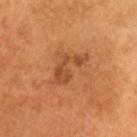workup: imaged on a skin check; not biopsied
patient: male, roughly 60 years of age
lesion diameter: ≈5 mm
location: the head or neck
lighting: cross-polarized illumination
acquisition: total-body-photography crop, ~15 mm field of view